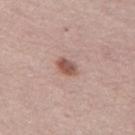The lesion was tiled from a total-body skin photograph and was not biopsied.
A female patient aged 63–67.
Automated tile analysis of the lesion measured an area of roughly 3.5 mm². The analysis additionally found a border-irregularity rating of about 1.5/10, a color-variation rating of about 2/10, and a peripheral color-asymmetry measure near 1. The software also gave an automated nevus-likeness rating near 90 out of 100 and a detector confidence of about 100 out of 100 that the crop contains a lesion.
This is a white-light tile.
Cropped from a total-body skin-imaging series; the visible field is about 15 mm.
The recorded lesion diameter is about 2.5 mm.
Located on the left thigh.